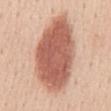Clinical impression:
The lesion was tiled from a total-body skin photograph and was not biopsied.
Background:
Longest diameter approximately 11 mm. The subject is a female aged 43 to 47. A region of skin cropped from a whole-body photographic capture, roughly 15 mm wide. The lesion is on the back.